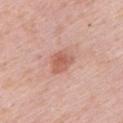Notes:
- notes: total-body-photography surveillance lesion; no biopsy
- illumination: white-light illumination
- lesion diameter: about 3 mm
- subject: female, approximately 45 years of age
- image source: total-body-photography crop, ~15 mm field of view
- body site: the left upper arm